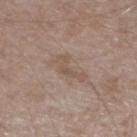The lesion was photographed on a routine skin check and not biopsied; there is no pathology result.
The lesion is located on the leg.
A lesion tile, about 15 mm wide, cut from a 3D total-body photograph.
A male patient in their mid- to late 40s.
The recorded lesion diameter is about 4 mm.
An algorithmic analysis of the crop reported a classifier nevus-likeness of about 0/100.